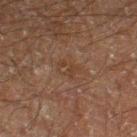Clinical impression: Imaged during a routine full-body skin examination; the lesion was not biopsied and no histopathology is available.